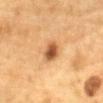follow-up: imaged on a skin check; not biopsied
patient: male, aged around 85 or older
imaging modality: ~15 mm crop, total-body skin-cancer survey
location: the chest